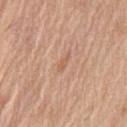<case>
<biopsy_status>not biopsied; imaged during a skin examination</biopsy_status>
<image>
  <source>total-body photography crop</source>
  <field_of_view_mm>15</field_of_view_mm>
</image>
<site>lower back</site>
<patient>
  <sex>male</sex>
  <age_approx>70</age_approx>
</patient>
<lesion_size>
  <long_diameter_mm_approx>2.5</long_diameter_mm_approx>
</lesion_size>
</case>